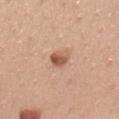Imaged during a routine full-body skin examination; the lesion was not biopsied and no histopathology is available.
The tile uses white-light illumination.
The lesion is on the back.
Cropped from a whole-body photographic skin survey; the tile spans about 15 mm.
A male subject aged 58–62.
Approximately 2.5 mm at its widest.